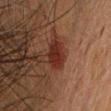This lesion was catalogued during total-body skin photography and was not selected for biopsy.
This image is a 15 mm lesion crop taken from a total-body photograph.
Automated image analysis of the tile measured an average lesion color of about L≈23 a*≈21 b*≈23 (CIELAB), a lesion–skin lightness drop of about 9, and a lesion-to-skin contrast of about 10 (normalized; higher = more distinct). And it measured a border-irregularity index near 2/10 and internal color variation of about 2.5 on a 0–10 scale.
The lesion's longest dimension is about 3.5 mm.
On the head or neck.
A female subject in their mid- to late 50s.
Captured under cross-polarized illumination.